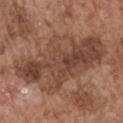Q: Was a biopsy performed?
A: no biopsy performed (imaged during a skin exam)
Q: What are the patient's age and sex?
A: male, aged 73–77
Q: Automated lesion metrics?
A: an average lesion color of about L≈44 a*≈20 b*≈27 (CIELAB) and roughly 10 lightness units darker than nearby skin; a nevus-likeness score of about 0/100 and a lesion-detection confidence of about 80/100
Q: What is the imaging modality?
A: ~15 mm crop, total-body skin-cancer survey
Q: Illumination type?
A: white-light
Q: What is the anatomic site?
A: the right upper arm